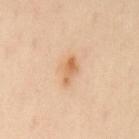Clinical impression:
Part of a total-body skin-imaging series; this lesion was reviewed on a skin check and was not flagged for biopsy.
Acquisition and patient details:
The subject is a female aged 53–57. A 15 mm close-up tile from a total-body photography series done for melanoma screening. The lesion's longest dimension is about 3.5 mm. This is a cross-polarized tile. On the upper back.